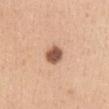Impression: Imaged during a routine full-body skin examination; the lesion was not biopsied and no histopathology is available. Context: The patient is a female in their 30s. A close-up tile cropped from a whole-body skin photograph, about 15 mm across. The lesion-visualizer software estimated a footprint of about 5.5 mm² and an outline eccentricity of about 0.6 (0 = round, 1 = elongated). The analysis additionally found a lesion-detection confidence of about 100/100. On the chest. Approximately 3 mm at its widest.